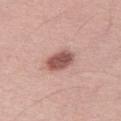A female subject, aged around 65.
A 15 mm close-up extracted from a 3D total-body photography capture.
The lesion is located on the right thigh.